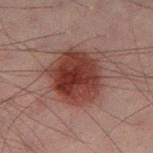Recorded during total-body skin imaging; not selected for excision or biopsy.
Approximately 6.5 mm at its widest.
Automated image analysis of the tile measured an average lesion color of about L≈30 a*≈20 b*≈20 (CIELAB) and a lesion-to-skin contrast of about 11 (normalized; higher = more distinct).
This image is a 15 mm lesion crop taken from a total-body photograph.
The lesion is located on the left thigh.
A male patient in their 40s.